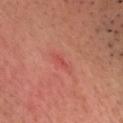Cropped from a whole-body photographic skin survey; the tile spans about 15 mm. The recorded lesion diameter is about 1.5 mm. The lesion is on the head or neck. A male patient, aged 53 to 57. Automated image analysis of the tile measured an average lesion color of about L≈48 a*≈35 b*≈30 (CIELAB), roughly 6 lightness units darker than nearby skin, and a normalized lesion–skin contrast near 4.5. It also reported an automated nevus-likeness rating near 0 out of 100. Histopathologically confirmed as an actinic keratosis (borderline).